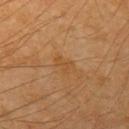workup: no biopsy performed (imaged during a skin exam)
subject: male, roughly 70 years of age
anatomic site: the left upper arm
acquisition: ~15 mm tile from a whole-body skin photo